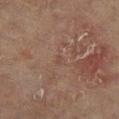Recorded during total-body skin imaging; not selected for excision or biopsy.
Captured under cross-polarized illumination.
Longest diameter approximately 1 mm.
A 15 mm close-up tile from a total-body photography series done for melanoma screening.
The lesion is located on the left lower leg.
A female subject aged approximately 80.
Automated image analysis of the tile measured a lesion area of about 1 mm² and two-axis asymmetry of about 0.5. The analysis additionally found a lesion color around L≈38 a*≈16 b*≈22 in CIELAB and a normalized lesion–skin contrast near 3.5.